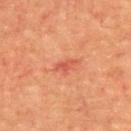Findings:
- follow-up — no biopsy performed (imaged during a skin exam)
- body site — the upper back
- diameter — about 3 mm
- illumination — cross-polarized
- imaging modality — ~15 mm crop, total-body skin-cancer survey
- patient — male, aged approximately 55